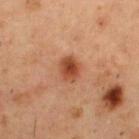The lesion was photographed on a routine skin check and not biopsied; there is no pathology result. Longest diameter approximately 3 mm. A male patient in their mid-50s. This is a cross-polarized tile. The total-body-photography lesion software estimated a mean CIELAB color near L≈47 a*≈27 b*≈35, roughly 13 lightness units darker than nearby skin, and a normalized lesion–skin contrast near 9.5. And it measured border irregularity of about 1.5 on a 0–10 scale and a color-variation rating of about 4.5/10. Located on the upper back. A lesion tile, about 15 mm wide, cut from a 3D total-body photograph.